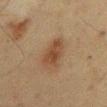A region of skin cropped from a whole-body photographic capture, roughly 15 mm wide. Located on the chest. Measured at roughly 4 mm in maximum diameter. A male subject, roughly 60 years of age.